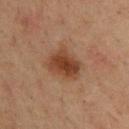Assessment: Imaged during a routine full-body skin examination; the lesion was not biopsied and no histopathology is available. Context: Approximately 4 mm at its widest. Located on the upper back. A lesion tile, about 15 mm wide, cut from a 3D total-body photograph. A male patient, in their mid- to late 30s.